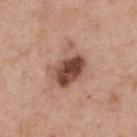Case summary:
* workup: total-body-photography surveillance lesion; no biopsy
* image source: 15 mm crop, total-body photography
* automated lesion analysis: an area of roughly 13 mm², an eccentricity of roughly 0.65, and a shape-asymmetry score of about 0.35 (0 = symmetric); a border-irregularity rating of about 4.5/10, internal color variation of about 8.5 on a 0–10 scale, and peripheral color asymmetry of about 3; a classifier nevus-likeness of about 55/100 and lesion-presence confidence of about 100/100
* anatomic site: the upper back
* subject: male, aged around 55